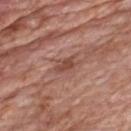Q: Was this lesion biopsied?
A: catalogued during a skin exam; not biopsied
Q: What is the lesion's diameter?
A: ≈3 mm
Q: How was the tile lit?
A: white-light
Q: What is the imaging modality?
A: 15 mm crop, total-body photography
Q: Patient demographics?
A: male, about 60 years old
Q: What is the anatomic site?
A: the chest